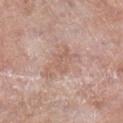<tbp_lesion>
  <biopsy_status>not biopsied; imaged during a skin examination</biopsy_status>
  <image>
    <source>total-body photography crop</source>
    <field_of_view_mm>15</field_of_view_mm>
  </image>
  <lighting>white-light</lighting>
  <lesion_size>
    <long_diameter_mm_approx>3.0</long_diameter_mm_approx>
  </lesion_size>
  <site>right lower leg</site>
  <patient>
    <sex>male</sex>
    <age_approx>75</age_approx>
  </patient>
</tbp_lesion>